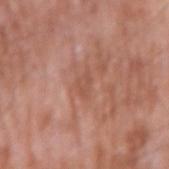| feature | finding |
|---|---|
| workup | total-body-photography surveillance lesion; no biopsy |
| patient | male, approximately 60 years of age |
| anatomic site | the right upper arm |
| diameter | ≈3 mm |
| tile lighting | white-light |
| acquisition | ~15 mm crop, total-body skin-cancer survey |